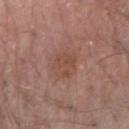{"biopsy_status": "not biopsied; imaged during a skin examination", "image": {"source": "total-body photography crop", "field_of_view_mm": 15}, "site": "right forearm", "patient": {"sex": "male", "age_approx": 70}, "lesion_size": {"long_diameter_mm_approx": 4.0}}